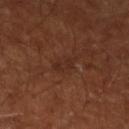notes: no biopsy performed (imaged during a skin exam) | site: the left lower leg | patient: aged approximately 65 | acquisition: 15 mm crop, total-body photography | TBP lesion metrics: a lesion color around L≈27 a*≈20 b*≈25 in CIELAB, roughly 5 lightness units darker than nearby skin, and a lesion-to-skin contrast of about 5 (normalized; higher = more distinct); a border-irregularity index near 4/10, a within-lesion color-variation index near 0/10, and radial color variation of about 0; an automated nevus-likeness rating near 0 out of 100 and lesion-presence confidence of about 100/100 | lighting: cross-polarized.